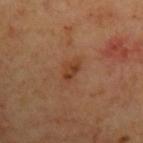Assessment: The lesion was tiled from a total-body skin photograph and was not biopsied. Clinical summary: A 15 mm close-up tile from a total-body photography series done for melanoma screening. A male subject approximately 70 years of age. From the left upper arm. Imaged with cross-polarized lighting. About 2.5 mm across.